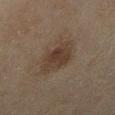{"biopsy_status": "not biopsied; imaged during a skin examination", "lighting": "cross-polarized", "site": "right lower leg", "patient": {"sex": "female", "age_approx": 60}, "lesion_size": {"long_diameter_mm_approx": 5.0}, "automated_metrics": {"area_mm2_approx": 13.0, "eccentricity": 0.75, "shape_asymmetry": 0.25, "cielab_L": 34, "cielab_a": 13, "cielab_b": 23, "vs_skin_darker_L": 7.0, "vs_skin_contrast_norm": 7.5, "border_irregularity_0_10": 3.0, "color_variation_0_10": 3.5, "peripheral_color_asymmetry": 1.0}, "image": {"source": "total-body photography crop", "field_of_view_mm": 15}}